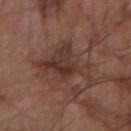Clinical impression: No biopsy was performed on this lesion — it was imaged during a full skin examination and was not determined to be concerning. Acquisition and patient details: A 15 mm close-up tile from a total-body photography series done for melanoma screening. The recorded lesion diameter is about 9 mm. The total-body-photography lesion software estimated a mean CIELAB color near L≈35 a*≈18 b*≈23, roughly 9 lightness units darker than nearby skin, and a normalized border contrast of about 8. It also reported a classifier nevus-likeness of about 5/100 and a detector confidence of about 60 out of 100 that the crop contains a lesion. The tile uses white-light illumination. A male patient, in their mid- to late 60s. From the arm.